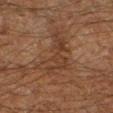• acquisition: ~15 mm crop, total-body skin-cancer survey
• automated metrics: a nevus-likeness score of about 0/100 and lesion-presence confidence of about 65/100
• site: the leg
• subject: male, roughly 60 years of age
• size: about 6 mm